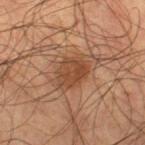Case summary:
• follow-up: catalogued during a skin exam; not biopsied